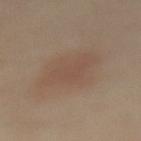Part of a total-body skin-imaging series; this lesion was reviewed on a skin check and was not flagged for biopsy.
Measured at roughly 5.5 mm in maximum diameter.
A female patient, aged 58–62.
An algorithmic analysis of the crop reported a mean CIELAB color near L≈50 a*≈15 b*≈26, a lesion–skin lightness drop of about 5, and a lesion-to-skin contrast of about 4 (normalized; higher = more distinct). The analysis additionally found a border-irregularity index near 2.5/10, a color-variation rating of about 2/10, and a peripheral color-asymmetry measure near 0.5.
The lesion is located on the front of the torso.
This image is a 15 mm lesion crop taken from a total-body photograph.